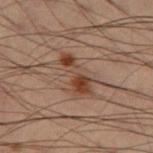Findings:
– workup · total-body-photography surveillance lesion; no biopsy
– site · the left thigh
– lesion diameter · about 5 mm
– patient · male, in their mid- to late 50s
– image source · 15 mm crop, total-body photography